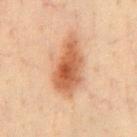Findings:
– notes: imaged on a skin check; not biopsied
– site: the chest
– illumination: cross-polarized illumination
– image source: 15 mm crop, total-body photography
– subject: male, about 35 years old